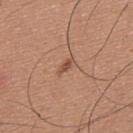notes=catalogued during a skin exam; not biopsied
subject=male, aged 33 to 37
diameter=about 2.5 mm
body site=the back
illumination=white-light
TBP lesion metrics=a shape eccentricity near 0.9 and two-axis asymmetry of about 0.2; an average lesion color of about L≈52 a*≈22 b*≈30 (CIELAB) and roughly 9 lightness units darker than nearby skin; a border-irregularity rating of about 2.5/10 and a within-lesion color-variation index near 1/10; a detector confidence of about 100 out of 100 that the crop contains a lesion
image source=~15 mm crop, total-body skin-cancer survey